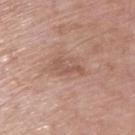biopsy status=catalogued during a skin exam; not biopsied
anatomic site=the upper back
patient=male, aged 63–67
acquisition=total-body-photography crop, ~15 mm field of view